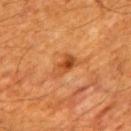No biopsy was performed on this lesion — it was imaged during a full skin examination and was not determined to be concerning. The recorded lesion diameter is about 3.5 mm. Cropped from a total-body skin-imaging series; the visible field is about 15 mm. Located on the mid back. A male subject, about 60 years old. The tile uses cross-polarized illumination.